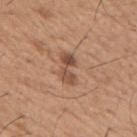Q: Was a biopsy performed?
A: no biopsy performed (imaged during a skin exam)
Q: Lesion size?
A: ≈3.5 mm
Q: What is the imaging modality?
A: ~15 mm crop, total-body skin-cancer survey
Q: Lesion location?
A: the right upper arm
Q: Who is the patient?
A: male, aged 63–67